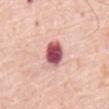Assessment: This lesion was catalogued during total-body skin photography and was not selected for biopsy. Background: Located on the mid back. A region of skin cropped from a whole-body photographic capture, roughly 15 mm wide. A male subject, aged approximately 80.